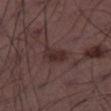Imaged during a routine full-body skin examination; the lesion was not biopsied and no histopathology is available. The lesion is located on the leg. About 3 mm across. A male patient aged around 50. Cropped from a total-body skin-imaging series; the visible field is about 15 mm. The lesion-visualizer software estimated a border-irregularity index near 2.5/10, a color-variation rating of about 2.5/10, and peripheral color asymmetry of about 1.